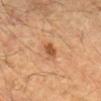workup = imaged on a skin check; not biopsied
body site = the left forearm
image = 15 mm crop, total-body photography
lighting = cross-polarized
subject = male, aged 83 or older
lesion diameter = ~3 mm (longest diameter)